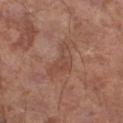Q: Was a biopsy performed?
A: total-body-photography surveillance lesion; no biopsy
Q: How large is the lesion?
A: ≈5 mm
Q: What are the patient's age and sex?
A: male, aged around 70
Q: Where on the body is the lesion?
A: the leg
Q: How was this image acquired?
A: ~15 mm tile from a whole-body skin photo
Q: What did automated image analysis measure?
A: an area of roughly 7 mm²; a lesion color around L≈47 a*≈21 b*≈28 in CIELAB, a lesion–skin lightness drop of about 6, and a lesion-to-skin contrast of about 5 (normalized; higher = more distinct); border irregularity of about 6 on a 0–10 scale, a within-lesion color-variation index near 3/10, and radial color variation of about 1; a nevus-likeness score of about 0/100 and lesion-presence confidence of about 100/100